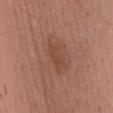workup: catalogued during a skin exam; not biopsied | imaging modality: 15 mm crop, total-body photography | site: the mid back | subject: female, aged 48 to 52 | illumination: white-light | diameter: ≈3.5 mm.